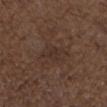Captured under white-light illumination. From the right forearm. A male patient, aged around 50. A 15 mm close-up extracted from a 3D total-body photography capture.